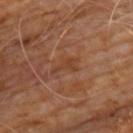<record>
  <biopsy_status>not biopsied; imaged during a skin examination</biopsy_status>
  <site>chest</site>
  <patient>
    <sex>male</sex>
    <age_approx>60</age_approx>
  </patient>
  <automated_metrics>
    <area_mm2_approx>3.0</area_mm2_approx>
    <eccentricity>0.85</eccentricity>
    <shape_asymmetry>0.6</shape_asymmetry>
    <peripheral_color_asymmetry>0.0</peripheral_color_asymmetry>
  </automated_metrics>
  <image>
    <source>total-body photography crop</source>
    <field_of_view_mm>15</field_of_view_mm>
  </image>
  <lesion_size>
    <long_diameter_mm_approx>2.5</long_diameter_mm_approx>
  </lesion_size>
</record>